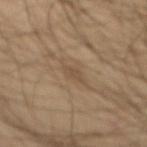Q: Was a biopsy performed?
A: total-body-photography surveillance lesion; no biopsy
Q: Where on the body is the lesion?
A: the mid back
Q: Who is the patient?
A: male, aged 58 to 62
Q: What kind of image is this?
A: ~15 mm crop, total-body skin-cancer survey